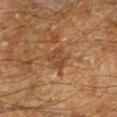biopsy status — total-body-photography surveillance lesion; no biopsy
tile lighting — cross-polarized illumination
location — the leg
image — ~15 mm crop, total-body skin-cancer survey
TBP lesion metrics — a lesion color around L≈34 a*≈17 b*≈27 in CIELAB and a normalized lesion–skin contrast near 6; a border-irregularity rating of about 4/10; a lesion-detection confidence of about 60/100
lesion size — ≈3 mm
subject — male, approximately 60 years of age